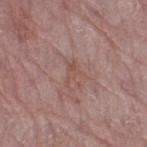| field | value |
|---|---|
| lesion size | ~3 mm (longest diameter) |
| site | the right thigh |
| patient | female, in their mid- to late 60s |
| TBP lesion metrics | a footprint of about 4.5 mm², an outline eccentricity of about 0.75 (0 = round, 1 = elongated), and a shape-asymmetry score of about 0.5 (0 = symmetric); a classifier nevus-likeness of about 0/100 and lesion-presence confidence of about 90/100 |
| tile lighting | white-light |
| image source | 15 mm crop, total-body photography |